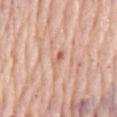{
  "biopsy_status": "not biopsied; imaged during a skin examination",
  "site": "chest",
  "lesion_size": {
    "long_diameter_mm_approx": 3.0
  },
  "lighting": "white-light",
  "automated_metrics": {
    "area_mm2_approx": 3.0,
    "eccentricity": 0.9,
    "border_irregularity_0_10": 4.0,
    "color_variation_0_10": 0.5,
    "peripheral_color_asymmetry": 0.0
  },
  "patient": {
    "sex": "male",
    "age_approx": 80
  },
  "image": {
    "source": "total-body photography crop",
    "field_of_view_mm": 15
  }
}